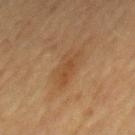On the mid back. A female subject approximately 60 years of age. The lesion-visualizer software estimated a border-irregularity index near 4.5/10 and peripheral color asymmetry of about 1. It also reported a lesion-detection confidence of about 100/100. A region of skin cropped from a whole-body photographic capture, roughly 15 mm wide. Imaged with cross-polarized lighting. Approximately 4 mm at its widest.